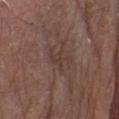| key | value |
|---|---|
| biopsy status | catalogued during a skin exam; not biopsied |
| lighting | white-light illumination |
| image-analysis metrics | an area of roughly 5 mm², a shape eccentricity near 0.75, and two-axis asymmetry of about 0.55; a border-irregularity rating of about 8.5/10, a color-variation rating of about 2/10, and peripheral color asymmetry of about 1 |
| subject | male, about 80 years old |
| location | the head or neck |
| acquisition | total-body-photography crop, ~15 mm field of view |